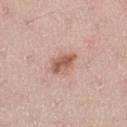<tbp_lesion>
<image>
  <source>total-body photography crop</source>
  <field_of_view_mm>15</field_of_view_mm>
</image>
<patient>
  <sex>female</sex>
  <age_approx>40</age_approx>
</patient>
<site>right thigh</site>
<lighting>white-light</lighting>
<lesion_size>
  <long_diameter_mm_approx>3.0</long_diameter_mm_approx>
</lesion_size>
</tbp_lesion>